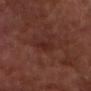The lesion was photographed on a routine skin check and not biopsied; there is no pathology result.
A close-up tile cropped from a whole-body skin photograph, about 15 mm across.
The tile uses cross-polarized illumination.
The lesion-visualizer software estimated an outline eccentricity of about 0.9 (0 = round, 1 = elongated) and two-axis asymmetry of about 0.2. The analysis additionally found a lesion color around L≈24 a*≈22 b*≈22 in CIELAB, roughly 5 lightness units darker than nearby skin, and a normalized lesion–skin contrast near 6. It also reported an automated nevus-likeness rating near 0 out of 100.
Located on the head or neck.
The lesion's longest dimension is about 2.5 mm.
A male patient, aged around 65.